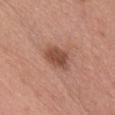Assessment:
This lesion was catalogued during total-body skin photography and was not selected for biopsy.
Clinical summary:
Located on the front of the torso. The patient is a female aged 38–42. The total-body-photography lesion software estimated a footprint of about 7 mm², an outline eccentricity of about 0.75 (0 = round, 1 = elongated), and two-axis asymmetry of about 0.25. It also reported a lesion color around L≈48 a*≈24 b*≈29 in CIELAB, a lesion–skin lightness drop of about 12, and a normalized border contrast of about 8.5. The analysis additionally found a border-irregularity rating of about 2.5/10 and a within-lesion color-variation index near 3.5/10. The recorded lesion diameter is about 3.5 mm. A 15 mm close-up tile from a total-body photography series done for melanoma screening.